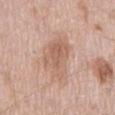Case summary:
* follow-up: catalogued during a skin exam; not biopsied
* imaging modality: ~15 mm tile from a whole-body skin photo
* tile lighting: white-light illumination
* location: the mid back
* lesion diameter: ≈6 mm
* subject: male, aged 58 to 62